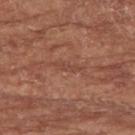Clinical impression: Captured during whole-body skin photography for melanoma surveillance; the lesion was not biopsied. Image and clinical context: Automated tile analysis of the lesion measured a mean CIELAB color near L≈45 a*≈24 b*≈28. The analysis additionally found border irregularity of about 5 on a 0–10 scale, internal color variation of about 0 on a 0–10 scale, and peripheral color asymmetry of about 0. It also reported an automated nevus-likeness rating near 0 out of 100 and a detector confidence of about 65 out of 100 that the crop contains a lesion. On the left forearm. A 15 mm crop from a total-body photograph taken for skin-cancer surveillance. A female patient aged 73–77.